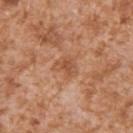<tbp_lesion>
<biopsy_status>not biopsied; imaged during a skin examination</biopsy_status>
<lesion_size>
  <long_diameter_mm_approx>3.0</long_diameter_mm_approx>
</lesion_size>
<image>
  <source>total-body photography crop</source>
  <field_of_view_mm>15</field_of_view_mm>
</image>
<patient>
  <sex>male</sex>
  <age_approx>45</age_approx>
</patient>
<lighting>white-light</lighting>
<site>right upper arm</site>
</tbp_lesion>